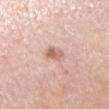follow-up: catalogued during a skin exam; not biopsied
lighting: white-light illumination
diameter: about 2.5 mm
automated metrics: a lesion area of about 4 mm², an eccentricity of roughly 0.6, and a shape-asymmetry score of about 0.25 (0 = symmetric); a border-irregularity rating of about 2.5/10, a within-lesion color-variation index near 4/10, and a peripheral color-asymmetry measure near 1.5; an automated nevus-likeness rating near 60 out of 100
image: ~15 mm crop, total-body skin-cancer survey
location: the right upper arm
subject: female, approximately 60 years of age